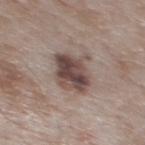| feature | finding |
|---|---|
| workup | imaged on a skin check; not biopsied |
| acquisition | 15 mm crop, total-body photography |
| illumination | white-light illumination |
| body site | the upper back |
| TBP lesion metrics | an average lesion color of about L≈46 a*≈15 b*≈19 (CIELAB) and a lesion-to-skin contrast of about 10 (normalized; higher = more distinct); a nevus-likeness score of about 60/100 and a detector confidence of about 100 out of 100 that the crop contains a lesion |
| patient | male, roughly 55 years of age |